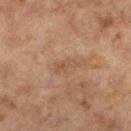biopsy status: catalogued during a skin exam; not biopsied | body site: the left lower leg | patient: female, aged 58–62 | imaging modality: 15 mm crop, total-body photography.